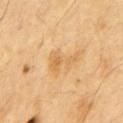– follow-up: imaged on a skin check; not biopsied
– lighting: cross-polarized illumination
– subject: male, aged around 70
– acquisition: ~15 mm crop, total-body skin-cancer survey
– anatomic site: the chest